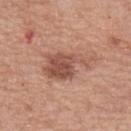Part of a total-body skin-imaging series; this lesion was reviewed on a skin check and was not flagged for biopsy. The patient is a female about 60 years old. On the arm. A 15 mm close-up extracted from a 3D total-body photography capture. Automated tile analysis of the lesion measured a footprint of about 14 mm² and a shape eccentricity near 0.85.